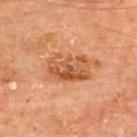Q: Was a biopsy performed?
A: imaged on a skin check; not biopsied
Q: Illumination type?
A: cross-polarized illumination
Q: What is the imaging modality?
A: ~15 mm tile from a whole-body skin photo
Q: Lesion size?
A: ≈5 mm
Q: Patient demographics?
A: male, approximately 65 years of age
Q: Automated lesion metrics?
A: a lesion area of about 13 mm² and an eccentricity of roughly 0.8; border irregularity of about 2 on a 0–10 scale, internal color variation of about 7.5 on a 0–10 scale, and peripheral color asymmetry of about 2.5; an automated nevus-likeness rating near 20 out of 100 and lesion-presence confidence of about 100/100
Q: What is the anatomic site?
A: the back